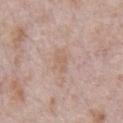notes: imaged on a skin check; not biopsied
acquisition: ~15 mm crop, total-body skin-cancer survey
site: the chest
lighting: white-light illumination
patient: male, aged 68 to 72